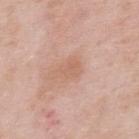follow-up = no biopsy performed (imaged during a skin exam) | automated lesion analysis = a footprint of about 5.5 mm²; about 6 CIELAB-L* units darker than the surrounding skin | size = ≈3 mm | anatomic site = the upper back | image source = ~15 mm crop, total-body skin-cancer survey | patient = male, in their mid- to late 50s | tile lighting = white-light.